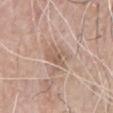Impression:
Recorded during total-body skin imaging; not selected for excision or biopsy.
Image and clinical context:
Located on the chest. The subject is a male aged approximately 80. Captured under white-light illumination. A 15 mm crop from a total-body photograph taken for skin-cancer surveillance.